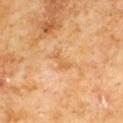follow-up: total-body-photography surveillance lesion; no biopsy
image source: ~15 mm tile from a whole-body skin photo
size: ≈3 mm
subject: male, aged approximately 60
body site: the front of the torso
tile lighting: cross-polarized illumination